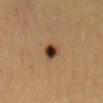notes = catalogued during a skin exam; not biopsied
subject = female, aged 38–42
illumination = cross-polarized
image = 15 mm crop, total-body photography
site = the mid back
size = about 2.5 mm